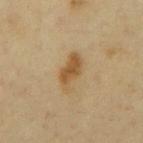biopsy status — total-body-photography surveillance lesion; no biopsy | automated lesion analysis — a border-irregularity index near 3.5/10, internal color variation of about 2.5 on a 0–10 scale, and radial color variation of about 1; an automated nevus-likeness rating near 80 out of 100 and a lesion-detection confidence of about 100/100 | acquisition — ~15 mm crop, total-body skin-cancer survey | subject — male, aged 63–67 | anatomic site — the chest | diameter — ≈4.5 mm.